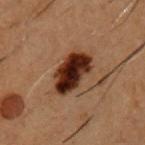subject: male, about 50 years old
imaging modality: ~15 mm crop, total-body skin-cancer survey
diameter: ~5.5 mm (longest diameter)
site: the chest
illumination: cross-polarized illumination
image-analysis metrics: a footprint of about 14 mm² and an outline eccentricity of about 0.85 (0 = round, 1 = elongated); a mean CIELAB color near L≈22 a*≈18 b*≈22, a lesion–skin lightness drop of about 17, and a lesion-to-skin contrast of about 16.5 (normalized; higher = more distinct); a classifier nevus-likeness of about 95/100 and a lesion-detection confidence of about 100/100